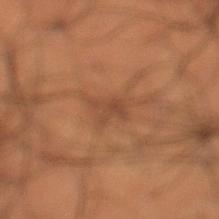<tbp_lesion>
  <biopsy_status>not biopsied; imaged during a skin examination</biopsy_status>
  <site>left lower leg</site>
  <lesion_size>
    <long_diameter_mm_approx>3.5</long_diameter_mm_approx>
  </lesion_size>
  <patient>
    <sex>male</sex>
    <age_approx>50</age_approx>
  </patient>
  <image>
    <source>total-body photography crop</source>
    <field_of_view_mm>15</field_of_view_mm>
  </image>
  <automated_metrics>
    <area_mm2_approx>4.0</area_mm2_approx>
    <shape_asymmetry>0.4</shape_asymmetry>
    <cielab_L>35</cielab_L>
    <cielab_a>18</cielab_a>
    <cielab_b>26</cielab_b>
    <vs_skin_darker_L>6.0</vs_skin_darker_L>
    <border_irregularity_0_10>5.0</border_irregularity_0_10>
    <peripheral_color_asymmetry>0.5</peripheral_color_asymmetry>
  </automated_metrics>
  <lighting>cross-polarized</lighting>
</tbp_lesion>